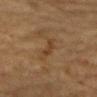• notes · imaged on a skin check; not biopsied
• patient · male, aged 83 or over
• body site · the mid back
• imaging modality · ~15 mm tile from a whole-body skin photo
• illumination · cross-polarized illumination
• lesion diameter · ~3 mm (longest diameter)